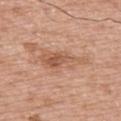Impression: No biopsy was performed on this lesion — it was imaged during a full skin examination and was not determined to be concerning. Context: This image is a 15 mm lesion crop taken from a total-body photograph. From the back. A male patient, in their mid-60s. Automated image analysis of the tile measured a lesion color around L≈57 a*≈22 b*≈32 in CIELAB and a normalized lesion–skin contrast near 6. And it measured a border-irregularity rating of about 5/10, internal color variation of about 6 on a 0–10 scale, and a peripheral color-asymmetry measure near 2.